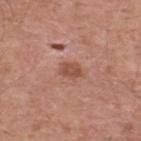Case summary:
• follow-up · catalogued during a skin exam; not biopsied
• diameter · ~2.5 mm (longest diameter)
• location · the upper back
• image source · 15 mm crop, total-body photography
• tile lighting · white-light illumination
• subject · male, roughly 55 years of age